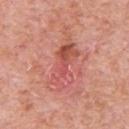Clinical impression:
The lesion was tiled from a total-body skin photograph and was not biopsied.
Clinical summary:
A 15 mm crop from a total-body photograph taken for skin-cancer surveillance. The recorded lesion diameter is about 6 mm. A male patient aged 78 to 82. Imaged with white-light lighting. The lesion is on the chest. The total-body-photography lesion software estimated a detector confidence of about 100 out of 100 that the crop contains a lesion.